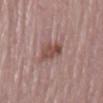{"biopsy_status": "not biopsied; imaged during a skin examination", "image": {"source": "total-body photography crop", "field_of_view_mm": 15}, "automated_metrics": {"cielab_L": 48, "cielab_a": 20, "cielab_b": 23, "vs_skin_darker_L": 10.0, "vs_skin_contrast_norm": 7.5, "border_irregularity_0_10": 2.5, "color_variation_0_10": 3.5, "peripheral_color_asymmetry": 1.5}, "lighting": "white-light", "site": "back", "lesion_size": {"long_diameter_mm_approx": 3.5}, "patient": {"sex": "male", "age_approx": "85+"}}